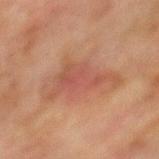| feature | finding |
|---|---|
| biopsy status | catalogued during a skin exam; not biopsied |
| image-analysis metrics | a lesion color around L≈43 a*≈22 b*≈26 in CIELAB and roughly 7 lightness units darker than nearby skin; border irregularity of about 6.5 on a 0–10 scale and a color-variation rating of about 3.5/10 |
| lesion size | about 7 mm |
| illumination | cross-polarized illumination |
| body site | the mid back |
| subject | male, aged 73–77 |
| image source | 15 mm crop, total-body photography |